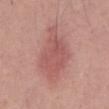The lesion was tiled from a total-body skin photograph and was not biopsied.
The lesion is located on the front of the torso.
About 7 mm across.
A male subject in their mid- to late 50s.
The lesion-visualizer software estimated a mean CIELAB color near L≈55 a*≈26 b*≈24 and a normalized lesion–skin contrast near 6. The analysis additionally found a nevus-likeness score of about 75/100 and lesion-presence confidence of about 100/100.
A lesion tile, about 15 mm wide, cut from a 3D total-body photograph.